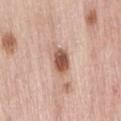The lesion was photographed on a routine skin check and not biopsied; there is no pathology result. Imaged with white-light lighting. A female subject approximately 65 years of age. The lesion is located on the lower back. The lesion's longest dimension is about 4 mm. A roughly 15 mm field-of-view crop from a total-body skin photograph.